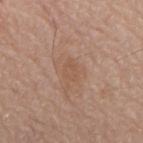| feature | finding |
|---|---|
| biopsy status | imaged on a skin check; not biopsied |
| image source | ~15 mm tile from a whole-body skin photo |
| image-analysis metrics | a lesion area of about 3.5 mm², a shape eccentricity near 0.7, and a shape-asymmetry score of about 0.25 (0 = symmetric); roughly 5 lightness units darker than nearby skin and a normalized lesion–skin contrast near 4.5 |
| anatomic site | the mid back |
| tile lighting | white-light illumination |
| subject | male, in their 80s |